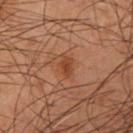Impression: Captured during whole-body skin photography for melanoma surveillance; the lesion was not biopsied. Clinical summary: A male subject in their mid- to late 50s. From the right forearm. Measured at roughly 2.5 mm in maximum diameter. This image is a 15 mm lesion crop taken from a total-body photograph.